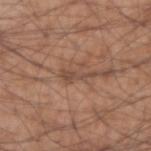acquisition = total-body-photography crop, ~15 mm field of view | patient = male, aged around 55 | location = the right forearm.